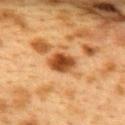Impression:
Captured during whole-body skin photography for melanoma surveillance; the lesion was not biopsied.
Clinical summary:
The lesion is located on the upper back. Longest diameter approximately 3.5 mm. A 15 mm crop from a total-body photograph taken for skin-cancer surveillance. The patient is a female roughly 40 years of age. This is a cross-polarized tile.